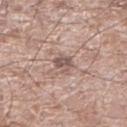| feature | finding |
|---|---|
| follow-up | total-body-photography surveillance lesion; no biopsy |